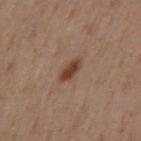{"biopsy_status": "not biopsied; imaged during a skin examination", "lesion_size": {"long_diameter_mm_approx": 3.0}, "patient": {"sex": "male", "age_approx": 40}, "site": "left upper arm", "image": {"source": "total-body photography crop", "field_of_view_mm": 15}}